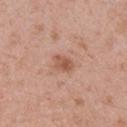notes: imaged on a skin check; not biopsied
image: 15 mm crop, total-body photography
tile lighting: white-light illumination
site: the right upper arm
patient: male, about 50 years old